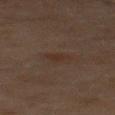Imaged during a routine full-body skin examination; the lesion was not biopsied and no histopathology is available. The lesion is on the mid back. The tile uses cross-polarized illumination. Cropped from a total-body skin-imaging series; the visible field is about 15 mm. Automated tile analysis of the lesion measured about 4 CIELAB-L* units darker than the surrounding skin and a lesion-to-skin contrast of about 5 (normalized; higher = more distinct). The software also gave a color-variation rating of about 1.5/10. About 2.5 mm across. A male patient, roughly 70 years of age.